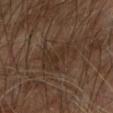Part of a total-body skin-imaging series; this lesion was reviewed on a skin check and was not flagged for biopsy.
The lesion is on the arm.
A 15 mm crop from a total-body photograph taken for skin-cancer surveillance.
A male subject in their 70s.